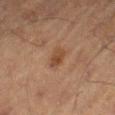The total-body-photography lesion software estimated a mean CIELAB color near L≈36 a*≈17 b*≈27, about 7 CIELAB-L* units darker than the surrounding skin, and a normalized border contrast of about 7. The software also gave border irregularity of about 2 on a 0–10 scale, internal color variation of about 3.5 on a 0–10 scale, and radial color variation of about 1.5. And it measured a nevus-likeness score of about 45/100. The recorded lesion diameter is about 3 mm. A lesion tile, about 15 mm wide, cut from a 3D total-body photograph. The lesion is located on the right thigh. A male patient, in their mid- to late 70s. The tile uses cross-polarized illumination.